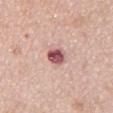workup = no biopsy performed (imaged during a skin exam) | diameter = ~3 mm (longest diameter) | subject = male, in their mid- to late 50s | image source = ~15 mm tile from a whole-body skin photo | TBP lesion metrics = a mean CIELAB color near L≈54 a*≈28 b*≈20, roughly 18 lightness units darker than nearby skin, and a normalized lesion–skin contrast near 11.5 | body site = the chest.